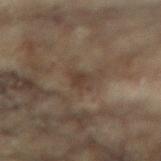– workup · imaged on a skin check; not biopsied
– lighting · cross-polarized illumination
– image source · 15 mm crop, total-body photography
– patient · female, aged around 80
– anatomic site · the arm
– lesion diameter · ~2.5 mm (longest diameter)